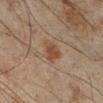The lesion was photographed on a routine skin check and not biopsied; there is no pathology result.
A male patient approximately 45 years of age.
The lesion is located on the right lower leg.
A 15 mm close-up tile from a total-body photography series done for melanoma screening.